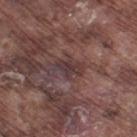biopsy status: imaged on a skin check; not biopsied
body site: the left thigh
imaging modality: total-body-photography crop, ~15 mm field of view
subject: male, approximately 75 years of age
lesion size: ~3 mm (longest diameter)
automated lesion analysis: an area of roughly 3.5 mm², an outline eccentricity of about 0.85 (0 = round, 1 = elongated), and a symmetry-axis asymmetry near 0.4; border irregularity of about 4 on a 0–10 scale, a within-lesion color-variation index near 1/10, and a peripheral color-asymmetry measure near 0; a detector confidence of about 55 out of 100 that the crop contains a lesion